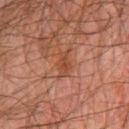Q: Was this lesion biopsied?
A: imaged on a skin check; not biopsied